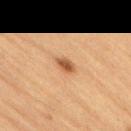Findings:
– biopsy status: no biopsy performed (imaged during a skin exam)
– subject: male, in their mid-80s or older
– location: the lower back
– imaging modality: total-body-photography crop, ~15 mm field of view